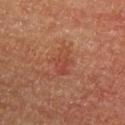This lesion was catalogued during total-body skin photography and was not selected for biopsy. The recorded lesion diameter is about 3.5 mm. The lesion is on the left upper arm. A roughly 15 mm field-of-view crop from a total-body skin photograph. A male patient, in their mid-60s. Automated tile analysis of the lesion measured a footprint of about 7 mm², an outline eccentricity of about 0.75 (0 = round, 1 = elongated), and a symmetry-axis asymmetry near 0.25. And it measured a lesion color around L≈47 a*≈28 b*≈32 in CIELAB and roughly 6 lightness units darker than nearby skin. Captured under cross-polarized illumination.